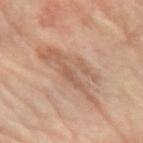Q: Where on the body is the lesion?
A: the left forearm
Q: How was the tile lit?
A: cross-polarized
Q: Patient demographics?
A: female, aged 63–67
Q: Lesion size?
A: ~8.5 mm (longest diameter)
Q: How was this image acquired?
A: ~15 mm tile from a whole-body skin photo